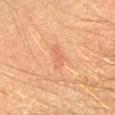Q: Was a biopsy performed?
A: no biopsy performed (imaged during a skin exam)
Q: What are the patient's age and sex?
A: male, aged 48–52
Q: What kind of image is this?
A: ~15 mm tile from a whole-body skin photo
Q: How was the tile lit?
A: cross-polarized
Q: Where on the body is the lesion?
A: the right forearm
Q: Automated lesion metrics?
A: a lesion area of about 3 mm², a shape eccentricity near 0.9, and two-axis asymmetry of about 0.5; internal color variation of about 0 on a 0–10 scale and peripheral color asymmetry of about 0; a nevus-likeness score of about 0/100 and a detector confidence of about 100 out of 100 that the crop contains a lesion